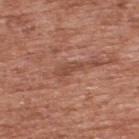  lighting: white-light
  site: back
  image:
    source: total-body photography crop
    field_of_view_mm: 15
  patient:
    sex: male
    age_approx: 70
  lesion_size:
    long_diameter_mm_approx: 3.5